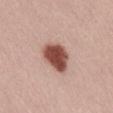No biopsy was performed on this lesion — it was imaged during a full skin examination and was not determined to be concerning.
A 15 mm close-up extracted from a 3D total-body photography capture.
About 4 mm across.
A male subject roughly 30 years of age.
The tile uses white-light illumination.